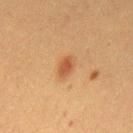Notes:
- workup: catalogued during a skin exam; not biopsied